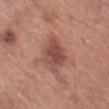Assessment: Imaged during a routine full-body skin examination; the lesion was not biopsied and no histopathology is available. Clinical summary: About 4.5 mm across. On the left thigh. A female subject aged around 75. This image is a 15 mm lesion crop taken from a total-body photograph. This is a white-light tile. An algorithmic analysis of the crop reported a shape eccentricity near 0.75 and two-axis asymmetry of about 0.2. The analysis additionally found a normalized border contrast of about 8.